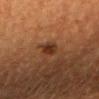Impression: Imaged during a routine full-body skin examination; the lesion was not biopsied and no histopathology is available. Acquisition and patient details: The recorded lesion diameter is about 2 mm. A close-up tile cropped from a whole-body skin photograph, about 15 mm across. On the head or neck. The tile uses cross-polarized illumination. A female subject, aged approximately 50.